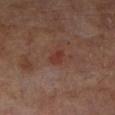Captured during whole-body skin photography for melanoma surveillance; the lesion was not biopsied. A close-up tile cropped from a whole-body skin photograph, about 15 mm across. An algorithmic analysis of the crop reported an average lesion color of about L≈34 a*≈24 b*≈24 (CIELAB) and roughly 6 lightness units darker than nearby skin. The analysis additionally found a classifier nevus-likeness of about 10/100 and a detector confidence of about 100 out of 100 that the crop contains a lesion. A male subject aged approximately 70. Imaged with cross-polarized lighting. The lesion's longest dimension is about 2.5 mm. From the right lower leg.